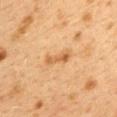Clinical impression: The lesion was tiled from a total-body skin photograph and was not biopsied. Acquisition and patient details: A female subject, aged 38 to 42. The lesion is located on the upper back. A roughly 15 mm field-of-view crop from a total-body skin photograph.